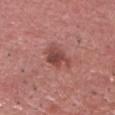Captured during whole-body skin photography for melanoma surveillance; the lesion was not biopsied.
Automated image analysis of the tile measured an eccentricity of roughly 0.7 and two-axis asymmetry of about 0.35. It also reported border irregularity of about 4.5 on a 0–10 scale. The analysis additionally found a nevus-likeness score of about 70/100 and a detector confidence of about 100 out of 100 that the crop contains a lesion.
Approximately 4 mm at its widest.
The tile uses white-light illumination.
A 15 mm close-up tile from a total-body photography series done for melanoma screening.
The subject is a male in their mid- to late 70s.
From the front of the torso.